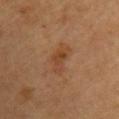The tile uses cross-polarized illumination.
The subject is a female about 60 years old.
The lesion is on the back.
A region of skin cropped from a whole-body photographic capture, roughly 15 mm wide.
Approximately 4 mm at its widest.
Automated tile analysis of the lesion measured an average lesion color of about L≈38 a*≈19 b*≈30 (CIELAB) and about 6 CIELAB-L* units darker than the surrounding skin. The software also gave a nevus-likeness score of about 65/100.